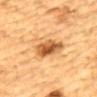The lesion was tiled from a total-body skin photograph and was not biopsied. From the upper back. Captured under cross-polarized illumination. A 15 mm close-up tile from a total-body photography series done for melanoma screening. A male subject, approximately 85 years of age or older.